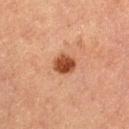Assessment: Imaged during a routine full-body skin examination; the lesion was not biopsied and no histopathology is available. Acquisition and patient details: The patient is a female approximately 60 years of age. The lesion is located on the left thigh. A 15 mm close-up tile from a total-body photography series done for melanoma screening. The lesion's longest dimension is about 2.5 mm. The tile uses cross-polarized illumination.